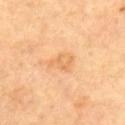{"biopsy_status": "not biopsied; imaged during a skin examination", "automated_metrics": {"area_mm2_approx": 4.0, "eccentricity": 0.85, "vs_skin_darker_L": 7.0, "vs_skin_contrast_norm": 5.0, "border_irregularity_0_10": 7.5, "color_variation_0_10": 0.0, "peripheral_color_asymmetry": 0.0}, "lesion_size": {"long_diameter_mm_approx": 3.0}, "patient": {"sex": "male", "age_approx": 70}, "lighting": "cross-polarized", "image": {"source": "total-body photography crop", "field_of_view_mm": 15}, "site": "upper back"}